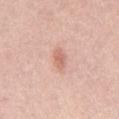Clinical impression:
No biopsy was performed on this lesion — it was imaged during a full skin examination and was not determined to be concerning.
Acquisition and patient details:
A male subject aged 38 to 42. An algorithmic analysis of the crop reported a lesion color around L≈64 a*≈25 b*≈29 in CIELAB, about 10 CIELAB-L* units darker than the surrounding skin, and a normalized border contrast of about 6.5. The software also gave a border-irregularity index near 3.5/10, internal color variation of about 1.5 on a 0–10 scale, and a peripheral color-asymmetry measure near 0.5. A 15 mm close-up extracted from a 3D total-body photography capture. The lesion is located on the chest. The lesion's longest dimension is about 2.5 mm.